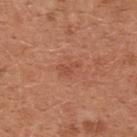Captured during whole-body skin photography for melanoma surveillance; the lesion was not biopsied. Located on the chest. Captured under white-light illumination. Longest diameter approximately 3 mm. A female patient, aged 33–37. A roughly 15 mm field-of-view crop from a total-body skin photograph.